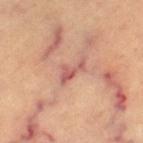Findings:
* biopsy status — no biopsy performed (imaged during a skin exam)
* automated metrics — a border-irregularity index near 6/10 and a within-lesion color-variation index near 2.5/10; an automated nevus-likeness rating near 0 out of 100
* lesion diameter — ~3.5 mm (longest diameter)
* tile lighting — cross-polarized
* anatomic site — the left thigh
* subject — roughly 60 years of age
* imaging modality — ~15 mm tile from a whole-body skin photo